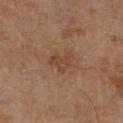Q: Is there a histopathology result?
A: total-body-photography surveillance lesion; no biopsy
Q: Automated lesion metrics?
A: an area of roughly 5.5 mm²; a classifier nevus-likeness of about 0/100 and a lesion-detection confidence of about 100/100
Q: What is the anatomic site?
A: the right lower leg
Q: How was the tile lit?
A: cross-polarized
Q: How was this image acquired?
A: 15 mm crop, total-body photography
Q: Patient demographics?
A: male, aged 63–67